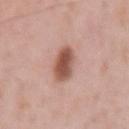workup: total-body-photography surveillance lesion; no biopsy | location: the left upper arm | imaging modality: ~15 mm tile from a whole-body skin photo | subject: male, about 50 years old.